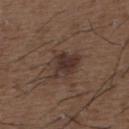The lesion was photographed on a routine skin check and not biopsied; there is no pathology result. Located on the back. Longest diameter approximately 4 mm. The subject is a male aged 48 to 52. A 15 mm close-up extracted from a 3D total-body photography capture. An algorithmic analysis of the crop reported an average lesion color of about L≈33 a*≈15 b*≈20 (CIELAB) and a lesion-to-skin contrast of about 8.5 (normalized; higher = more distinct). The software also gave a border-irregularity rating of about 3/10 and a within-lesion color-variation index near 4/10. This is a white-light tile.